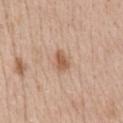Part of a total-body skin-imaging series; this lesion was reviewed on a skin check and was not flagged for biopsy. Captured under white-light illumination. A 15 mm crop from a total-body photograph taken for skin-cancer surveillance. The lesion is located on the abdomen. A female patient in their mid-40s.